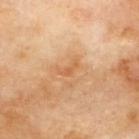Assessment: The lesion was tiled from a total-body skin photograph and was not biopsied. Acquisition and patient details: The patient is a male aged around 70. About 2.5 mm across. An algorithmic analysis of the crop reported an average lesion color of about L≈60 a*≈23 b*≈40 (CIELAB), a lesion–skin lightness drop of about 7, and a normalized border contrast of about 5.5. The software also gave internal color variation of about 0 on a 0–10 scale and radial color variation of about 0. Captured under cross-polarized illumination. This image is a 15 mm lesion crop taken from a total-body photograph. From the back.